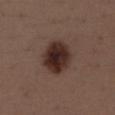This image is a 15 mm lesion crop taken from a total-body photograph. A female patient aged around 50. The lesion is located on the chest.